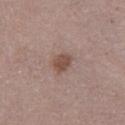Impression:
This lesion was catalogued during total-body skin photography and was not selected for biopsy.
Background:
The lesion is located on the mid back. The total-body-photography lesion software estimated an outline eccentricity of about 0.5 (0 = round, 1 = elongated) and two-axis asymmetry of about 0.2. This is a white-light tile. A female subject aged around 40. A region of skin cropped from a whole-body photographic capture, roughly 15 mm wide.